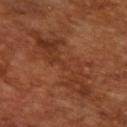Imaged with cross-polarized lighting. Automated tile analysis of the lesion measured an average lesion color of about L≈34 a*≈24 b*≈31 (CIELAB). And it measured border irregularity of about 8.5 on a 0–10 scale, a within-lesion color-variation index near 4/10, and radial color variation of about 1.5. The software also gave an automated nevus-likeness rating near 0 out of 100 and lesion-presence confidence of about 80/100. Cropped from a total-body skin-imaging series; the visible field is about 15 mm. The lesion's longest dimension is about 11.5 mm. The subject is a male approximately 65 years of age.